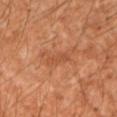notes: total-body-photography surveillance lesion; no biopsy | tile lighting: cross-polarized | TBP lesion metrics: a footprint of about 3 mm² | lesion diameter: about 3 mm | patient: male, in their mid- to late 50s | location: the left upper arm | imaging modality: ~15 mm crop, total-body skin-cancer survey.